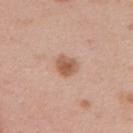Impression:
Part of a total-body skin-imaging series; this lesion was reviewed on a skin check and was not flagged for biopsy.
Image and clinical context:
A female patient in their 50s. A lesion tile, about 15 mm wide, cut from a 3D total-body photograph. The lesion is on the upper back.